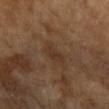Context: The subject is a female aged approximately 70. The lesion-visualizer software estimated a footprint of about 3.5 mm² and a shape-asymmetry score of about 0.3 (0 = symmetric). It also reported a lesion color around L≈34 a*≈17 b*≈28 in CIELAB, about 6 CIELAB-L* units darker than the surrounding skin, and a normalized border contrast of about 5.5. The analysis additionally found a border-irregularity index near 3/10, a within-lesion color-variation index near 1/10, and peripheral color asymmetry of about 0.5. The software also gave a nevus-likeness score of about 0/100 and a detector confidence of about 100 out of 100 that the crop contains a lesion. About 2.5 mm across. From the right forearm. Cropped from a whole-body photographic skin survey; the tile spans about 15 mm. This is a cross-polarized tile.